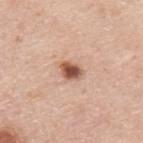The lesion was tiled from a total-body skin photograph and was not biopsied. The lesion is on the back. Cropped from a total-body skin-imaging series; the visible field is about 15 mm. A male patient, aged approximately 45.